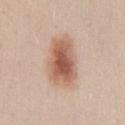Findings:
• notes: total-body-photography surveillance lesion; no biopsy
• acquisition: ~15 mm crop, total-body skin-cancer survey
• patient: male, aged approximately 30
• TBP lesion metrics: a lesion area of about 18 mm², an eccentricity of roughly 0.8, and two-axis asymmetry of about 0.2; a lesion color around L≈59 a*≈21 b*≈29 in CIELAB, a lesion–skin lightness drop of about 14, and a normalized lesion–skin contrast near 9
• lighting: white-light illumination
• location: the front of the torso